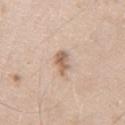Q: Lesion location?
A: the arm
Q: How was this image acquired?
A: ~15 mm tile from a whole-body skin photo
Q: Automated lesion metrics?
A: a shape eccentricity near 0.85 and two-axis asymmetry of about 0.3; a border-irregularity index near 3.5/10, internal color variation of about 3.5 on a 0–10 scale, and radial color variation of about 1
Q: Who is the patient?
A: male, in their mid- to late 20s